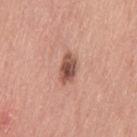{
  "biopsy_status": "not biopsied; imaged during a skin examination",
  "patient": {
    "sex": "female",
    "age_approx": 65
  },
  "site": "left thigh",
  "image": {
    "source": "total-body photography crop",
    "field_of_view_mm": 15
  }
}